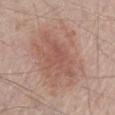Imaged during a routine full-body skin examination; the lesion was not biopsied and no histopathology is available.
The lesion is located on the front of the torso.
Cropped from a total-body skin-imaging series; the visible field is about 15 mm.
A male patient in their 70s.
The recorded lesion diameter is about 6.5 mm.